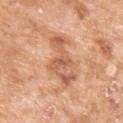<tbp_lesion>
  <biopsy_status>not biopsied; imaged during a skin examination</biopsy_status>
  <site>left upper arm</site>
  <automated_metrics>
    <nevus_likeness_0_100>0</nevus_likeness_0_100>
    <lesion_detection_confidence_0_100>100</lesion_detection_confidence_0_100>
  </automated_metrics>
  <image>
    <source>total-body photography crop</source>
    <field_of_view_mm>15</field_of_view_mm>
  </image>
  <lighting>white-light</lighting>
  <patient>
    <sex>female</sex>
    <age_approx>75</age_approx>
  </patient>
</tbp_lesion>